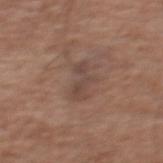Captured during whole-body skin photography for melanoma surveillance; the lesion was not biopsied.
The total-body-photography lesion software estimated a footprint of about 5.5 mm², a shape eccentricity near 0.9, and two-axis asymmetry of about 0.4. The software also gave border irregularity of about 6 on a 0–10 scale, a color-variation rating of about 1/10, and radial color variation of about 0.5.
Imaged with white-light lighting.
A region of skin cropped from a whole-body photographic capture, roughly 15 mm wide.
The lesion is on the upper back.
A male subject in their mid- to late 70s.
Measured at roughly 4 mm in maximum diameter.